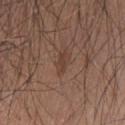Captured during whole-body skin photography for melanoma surveillance; the lesion was not biopsied. Automated image analysis of the tile measured a lesion area of about 3 mm², a shape eccentricity near 0.8, and two-axis asymmetry of about 0.3. The analysis additionally found a lesion color around L≈40 a*≈18 b*≈25 in CIELAB, roughly 6 lightness units darker than nearby skin, and a normalized lesion–skin contrast near 5.5. The patient is a male roughly 50 years of age. From the back. A 15 mm close-up extracted from a 3D total-body photography capture. Longest diameter approximately 2.5 mm. The tile uses white-light illumination.